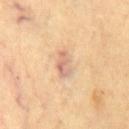Acquisition and patient details: A male subject about 70 years old. This is a cross-polarized tile. A 15 mm close-up tile from a total-body photography series done for melanoma screening. Located on the chest. Longest diameter approximately 3 mm.